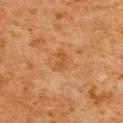Findings:
– follow-up — total-body-photography surveillance lesion; no biopsy
– patient — male, aged around 60
– automated metrics — a lesion color around L≈44 a*≈21 b*≈36 in CIELAB, about 6 CIELAB-L* units darker than the surrounding skin, and a lesion-to-skin contrast of about 5.5 (normalized; higher = more distinct); border irregularity of about 3 on a 0–10 scale, a within-lesion color-variation index near 1/10, and radial color variation of about 0.5; a nevus-likeness score of about 0/100 and a lesion-detection confidence of about 100/100
– acquisition — total-body-photography crop, ~15 mm field of view
– location — the back
– lighting — cross-polarized